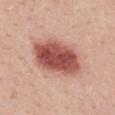notes — total-body-photography surveillance lesion; no biopsy | subject — male, in their mid- to late 20s | image — ~15 mm crop, total-body skin-cancer survey | anatomic site — the mid back.